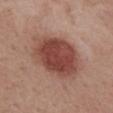The lesion was photographed on a routine skin check and not biopsied; there is no pathology result. A lesion tile, about 15 mm wide, cut from a 3D total-body photograph. The lesion is on the mid back. A male patient, in their mid- to late 50s. The tile uses white-light illumination.